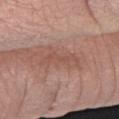<record>
  <biopsy_status>not biopsied; imaged during a skin examination</biopsy_status>
  <site>right forearm</site>
  <automated_metrics>
    <vs_skin_darker_L>7.0</vs_skin_darker_L>
    <vs_skin_contrast_norm>5.0</vs_skin_contrast_norm>
  </automated_metrics>
  <patient>
    <sex>male</sex>
    <age_approx>40</age_approx>
  </patient>
  <lesion_size>
    <long_diameter_mm_approx>5.0</long_diameter_mm_approx>
  </lesion_size>
  <image>
    <source>total-body photography crop</source>
    <field_of_view_mm>15</field_of_view_mm>
  </image>
</record>